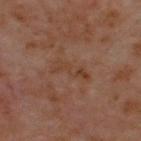<tbp_lesion>
<biopsy_status>not biopsied; imaged during a skin examination</biopsy_status>
<image>
  <source>total-body photography crop</source>
  <field_of_view_mm>15</field_of_view_mm>
</image>
<lighting>cross-polarized</lighting>
<patient>
  <sex>male</sex>
  <age_approx>60</age_approx>
</patient>
<lesion_size>
  <long_diameter_mm_approx>4.5</long_diameter_mm_approx>
</lesion_size>
<site>upper back</site>
</tbp_lesion>